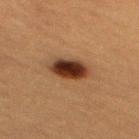biopsy status = no biopsy performed (imaged during a skin exam) | location = the mid back | patient = female, in their mid- to late 50s | tile lighting = cross-polarized illumination | automated lesion analysis = a lesion area of about 9.5 mm² and an eccentricity of roughly 0.75; a border-irregularity index near 1.5/10 and a peripheral color-asymmetry measure near 2; an automated nevus-likeness rating near 100 out of 100 | acquisition = ~15 mm tile from a whole-body skin photo.